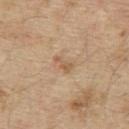Clinical impression: Recorded during total-body skin imaging; not selected for excision or biopsy.